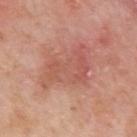Impression: The lesion was tiled from a total-body skin photograph and was not biopsied. Image and clinical context: Approximately 7 mm at its widest. A male patient in their mid-70s. Automated image analysis of the tile measured a lesion area of about 19 mm², an eccentricity of roughly 0.8, and a shape-asymmetry score of about 0.45 (0 = symmetric). It also reported an average lesion color of about L≈56 a*≈26 b*≈29 (CIELAB), a lesion–skin lightness drop of about 7, and a normalized border contrast of about 5. From the left upper arm. This image is a 15 mm lesion crop taken from a total-body photograph. Imaged with white-light lighting.